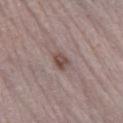| field | value |
|---|---|
| workup | total-body-photography surveillance lesion; no biopsy |
| lighting | white-light illumination |
| image | total-body-photography crop, ~15 mm field of view |
| anatomic site | the right lower leg |
| automated metrics | a lesion area of about 3.5 mm², a shape eccentricity near 0.7, and two-axis asymmetry of about 0.3; an average lesion color of about L≈47 a*≈17 b*≈21 (CIELAB), about 10 CIELAB-L* units darker than the surrounding skin, and a lesion-to-skin contrast of about 8.5 (normalized; higher = more distinct); a border-irregularity index near 2.5/10, a color-variation rating of about 3.5/10, and a peripheral color-asymmetry measure near 1.5; a nevus-likeness score of about 75/100 and a detector confidence of about 100 out of 100 that the crop contains a lesion |
| subject | male, about 70 years old |
| lesion diameter | ~2.5 mm (longest diameter) |